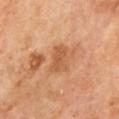The lesion was tiled from a total-body skin photograph and was not biopsied. The lesion's longest dimension is about 3 mm. A 15 mm close-up extracted from a 3D total-body photography capture. A male patient, aged around 70. Imaged with cross-polarized lighting. On the mid back. The lesion-visualizer software estimated a classifier nevus-likeness of about 0/100 and a detector confidence of about 100 out of 100 that the crop contains a lesion.